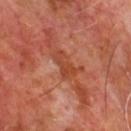Captured during whole-body skin photography for melanoma surveillance; the lesion was not biopsied.
On the chest.
A region of skin cropped from a whole-body photographic capture, roughly 15 mm wide.
A male patient aged around 65.
Approximately 4 mm at its widest.
This is a cross-polarized tile.
Automated image analysis of the tile measured a nevus-likeness score of about 0/100 and lesion-presence confidence of about 85/100.